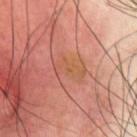biopsy_status: not biopsied; imaged during a skin examination
lighting: cross-polarized
image:
  source: total-body photography crop
  field_of_view_mm: 15
automated_metrics:
  area_mm2_approx: 6.5
  eccentricity: 0.85
  shape_asymmetry: 0.4
  cielab_L: 55
  cielab_a: 25
  cielab_b: 34
  vs_skin_darker_L: 5.0
  vs_skin_contrast_norm: 5.0
  border_irregularity_0_10: 5.0
  color_variation_0_10: 3.5
  peripheral_color_asymmetry: 1.0
site: chest
patient:
  sex: male
  age_approx: 50
lesion_size:
  long_diameter_mm_approx: 3.5